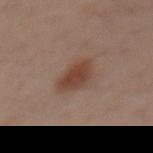Impression: The lesion was tiled from a total-body skin photograph and was not biopsied. Clinical summary: The lesion is located on the chest. A 15 mm close-up extracted from a 3D total-body photography capture. A male patient, aged 38–42.